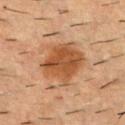notes — no biopsy performed (imaged during a skin exam) | image — ~15 mm crop, total-body skin-cancer survey | lesion size — ~5.5 mm (longest diameter) | patient — male, in their mid-50s | tile lighting — cross-polarized | anatomic site — the upper back.